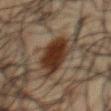Findings:
- anatomic site · the chest
- image · ~15 mm crop, total-body skin-cancer survey
- patient · male, roughly 60 years of age